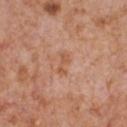Q: Was a biopsy performed?
A: catalogued during a skin exam; not biopsied
Q: Where on the body is the lesion?
A: the chest
Q: How was this image acquired?
A: ~15 mm crop, total-body skin-cancer survey
Q: Who is the patient?
A: male, roughly 65 years of age